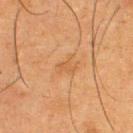No biopsy was performed on this lesion — it was imaged during a full skin examination and was not determined to be concerning.
Approximately 2.5 mm at its widest.
A male patient, in their mid- to late 30s.
This image is a 15 mm lesion crop taken from a total-body photograph.
This is a cross-polarized tile.
On the back.